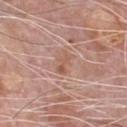<case>
<biopsy_status>not biopsied; imaged during a skin examination</biopsy_status>
<site>chest</site>
<image>
  <source>total-body photography crop</source>
  <field_of_view_mm>15</field_of_view_mm>
</image>
<lighting>white-light</lighting>
<patient>
  <sex>male</sex>
  <age_approx>60</age_approx>
</patient>
</case>